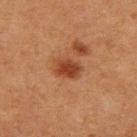Assessment:
This lesion was catalogued during total-body skin photography and was not selected for biopsy.
Acquisition and patient details:
A lesion tile, about 15 mm wide, cut from a 3D total-body photograph. The subject is a female roughly 50 years of age. Approximately 3 mm at its widest. Imaged with cross-polarized lighting. On the left thigh.